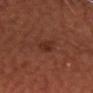This lesion was catalogued during total-body skin photography and was not selected for biopsy. A 15 mm close-up extracted from a 3D total-body photography capture. Located on the head or neck. Captured under cross-polarized illumination. A male subject, in their mid-60s.